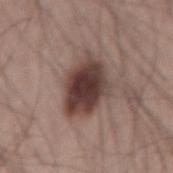{
  "biopsy_status": "not biopsied; imaged during a skin examination",
  "site": "left thigh",
  "lesion_size": {
    "long_diameter_mm_approx": 6.5
  },
  "patient": {
    "sex": "male",
    "age_approx": 50
  },
  "image": {
    "source": "total-body photography crop",
    "field_of_view_mm": 15
  },
  "lighting": "white-light"
}